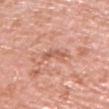| field | value |
|---|---|
| biopsy status | total-body-photography surveillance lesion; no biopsy |
| image | ~15 mm crop, total-body skin-cancer survey |
| location | the chest |
| subject | male, aged around 70 |